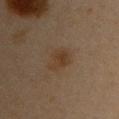Clinical impression:
Part of a total-body skin-imaging series; this lesion was reviewed on a skin check and was not flagged for biopsy.
Background:
The lesion is on the left upper arm. A 15 mm close-up tile from a total-body photography series done for melanoma screening. The patient is a female in their mid-40s.